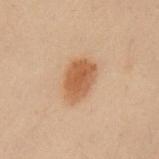The lesion was photographed on a routine skin check and not biopsied; there is no pathology result.
Cropped from a total-body skin-imaging series; the visible field is about 15 mm.
A male subject, approximately 55 years of age.
The tile uses cross-polarized illumination.
From the left upper arm.
The lesion-visualizer software estimated an average lesion color of about L≈46 a*≈18 b*≈30 (CIELAB), a lesion–skin lightness drop of about 10, and a lesion-to-skin contrast of about 8.5 (normalized; higher = more distinct). The software also gave a border-irregularity rating of about 2/10, internal color variation of about 2.5 on a 0–10 scale, and peripheral color asymmetry of about 1.